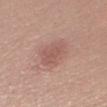Notes:
* notes — total-body-photography surveillance lesion; no biopsy
* diameter — ~5 mm (longest diameter)
* lighting — white-light illumination
* body site — the right thigh
* acquisition — 15 mm crop, total-body photography
* patient — female, roughly 60 years of age